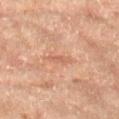Recorded during total-body skin imaging; not selected for excision or biopsy. A male subject, roughly 65 years of age. A close-up tile cropped from a whole-body skin photograph, about 15 mm across. The lesion is located on the right upper arm.